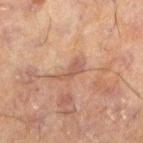No biopsy was performed on this lesion — it was imaged during a full skin examination and was not determined to be concerning. A lesion tile, about 15 mm wide, cut from a 3D total-body photograph. The lesion's longest dimension is about 4 mm. A male patient about 60 years old. The tile uses cross-polarized illumination. Located on the left lower leg.